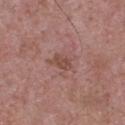notes: imaged on a skin check; not biopsied
location: the upper back
image source: ~15 mm tile from a whole-body skin photo
subject: male, approximately 70 years of age